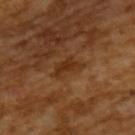illumination: cross-polarized illumination
automated lesion analysis: a footprint of about 6 mm² and a symmetry-axis asymmetry near 0.4; a border-irregularity index near 4.5/10, a within-lesion color-variation index near 2/10, and radial color variation of about 0.5
image: total-body-photography crop, ~15 mm field of view
patient: male, aged around 65